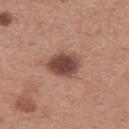notes: total-body-photography surveillance lesion; no biopsy
site: the left thigh
subject: female, approximately 30 years of age
image source: ~15 mm crop, total-body skin-cancer survey
illumination: white-light